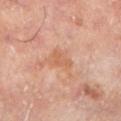The lesion was tiled from a total-body skin photograph and was not biopsied. Automated tile analysis of the lesion measured a lesion area of about 3.5 mm², an outline eccentricity of about 0.8 (0 = round, 1 = elongated), and a shape-asymmetry score of about 0.45 (0 = symmetric). The analysis additionally found a lesion–skin lightness drop of about 7. The analysis additionally found a lesion-detection confidence of about 100/100. This is a cross-polarized tile. Measured at roughly 2.5 mm in maximum diameter. From the leg. A lesion tile, about 15 mm wide, cut from a 3D total-body photograph. The subject is a male approximately 65 years of age.